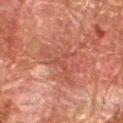workup: no biopsy performed (imaged during a skin exam); body site: the right forearm; patient: male, aged approximately 80; illumination: cross-polarized; image: ~15 mm tile from a whole-body skin photo.